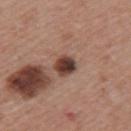Case summary:
• follow-up: total-body-photography surveillance lesion; no biopsy
• patient: female, aged 43–47
• site: the back
• lighting: white-light illumination
• automated metrics: a shape eccentricity near 0.65; a classifier nevus-likeness of about 95/100 and a lesion-detection confidence of about 100/100
• image: ~15 mm tile from a whole-body skin photo